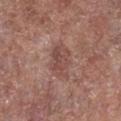follow-up: total-body-photography surveillance lesion; no biopsy | acquisition: ~15 mm crop, total-body skin-cancer survey | anatomic site: the right lower leg | image-analysis metrics: a shape-asymmetry score of about 0.25 (0 = symmetric) | diameter: ~4 mm (longest diameter) | lighting: white-light illumination | subject: male, roughly 55 years of age.